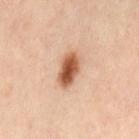Assessment: No biopsy was performed on this lesion — it was imaged during a full skin examination and was not determined to be concerning. Clinical summary: Imaged with cross-polarized lighting. The lesion is on the lower back. Cropped from a total-body skin-imaging series; the visible field is about 15 mm. The lesion's longest dimension is about 4.5 mm. A female patient, roughly 40 years of age.